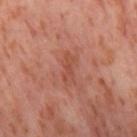{
  "biopsy_status": "not biopsied; imaged during a skin examination",
  "site": "right thigh",
  "lighting": "cross-polarized",
  "patient": {
    "sex": "female",
    "age_approx": 55
  },
  "image": {
    "source": "total-body photography crop",
    "field_of_view_mm": 15
  },
  "lesion_size": {
    "long_diameter_mm_approx": 3.0
  }
}